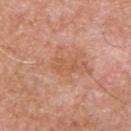Assessment: Captured during whole-body skin photography for melanoma surveillance; the lesion was not biopsied. Acquisition and patient details: Measured at roughly 3 mm in maximum diameter. Captured under white-light illumination. From the back. A lesion tile, about 15 mm wide, cut from a 3D total-body photograph. The subject is a male aged approximately 55.